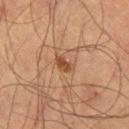Image and clinical context:
The lesion's longest dimension is about 2.5 mm. Automated image analysis of the tile measured an average lesion color of about L≈38 a*≈19 b*≈28 (CIELAB) and a lesion–skin lightness drop of about 9. It also reported a border-irregularity index near 2.5/10, a within-lesion color-variation index near 2/10, and peripheral color asymmetry of about 0.5. The analysis additionally found an automated nevus-likeness rating near 85 out of 100. A 15 mm close-up tile from a total-body photography series done for melanoma screening. A male subject aged around 65. This is a cross-polarized tile. From the left thigh.